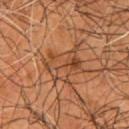Q: Was this lesion biopsied?
A: catalogued during a skin exam; not biopsied